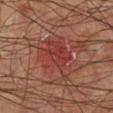Clinical impression:
Imaged during a routine full-body skin examination; the lesion was not biopsied and no histopathology is available.
Background:
Cropped from a whole-body photographic skin survey; the tile spans about 15 mm. Imaged with cross-polarized lighting. On the left upper arm. A male patient, about 75 years old. The recorded lesion diameter is about 6 mm.